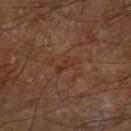Q: Is there a histopathology result?
A: imaged on a skin check; not biopsied
Q: Patient demographics?
A: male, about 70 years old
Q: What is the anatomic site?
A: the left forearm
Q: How was this image acquired?
A: ~15 mm tile from a whole-body skin photo
Q: What did automated image analysis measure?
A: an area of roughly 3 mm², a shape eccentricity near 0.9, and a symmetry-axis asymmetry near 0.35; an average lesion color of about L≈31 a*≈19 b*≈27 (CIELAB), roughly 5 lightness units darker than nearby skin, and a lesion-to-skin contrast of about 5 (normalized; higher = more distinct); a border-irregularity index near 4/10 and a color-variation rating of about 0/10; an automated nevus-likeness rating near 0 out of 100 and a lesion-detection confidence of about 95/100
Q: Illumination type?
A: cross-polarized